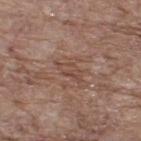tile lighting: white-light; subject: male, aged around 70; diameter: about 3.5 mm; body site: the back; image source: total-body-photography crop, ~15 mm field of view.